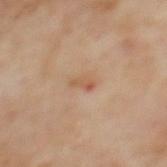Q: Was this lesion biopsied?
A: imaged on a skin check; not biopsied
Q: Lesion location?
A: the upper back
Q: What kind of image is this?
A: ~15 mm tile from a whole-body skin photo
Q: What are the patient's age and sex?
A: female, approximately 60 years of age